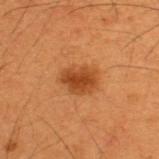Captured during whole-body skin photography for melanoma surveillance; the lesion was not biopsied. A male patient, in their 50s. Longest diameter approximately 3.5 mm. The lesion is located on the back. This is a cross-polarized tile. A 15 mm close-up extracted from a 3D total-body photography capture.